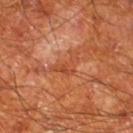Captured during whole-body skin photography for melanoma surveillance; the lesion was not biopsied. A male patient, aged approximately 65. Cropped from a whole-body photographic skin survey; the tile spans about 15 mm. The lesion is on the left lower leg.